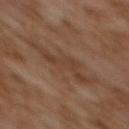A female patient roughly 60 years of age. This is a cross-polarized tile. On the upper back. The recorded lesion diameter is about 7.5 mm. This image is a 15 mm lesion crop taken from a total-body photograph.